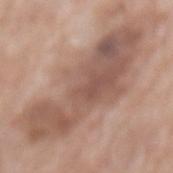Assessment: This lesion was catalogued during total-body skin photography and was not selected for biopsy. Clinical summary: From the mid back. A female subject approximately 75 years of age. Automated tile analysis of the lesion measured an area of roughly 48 mm², a shape eccentricity near 0.95, and two-axis asymmetry of about 0.35. It also reported a border-irregularity rating of about 5.5/10 and radial color variation of about 2. And it measured a nevus-likeness score of about 0/100 and lesion-presence confidence of about 95/100. This is a white-light tile. A close-up tile cropped from a whole-body skin photograph, about 15 mm across.